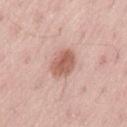biopsy status = no biopsy performed (imaged during a skin exam)
subject = male, about 55 years old
anatomic site = the lower back
imaging modality = total-body-photography crop, ~15 mm field of view